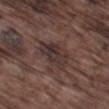workup: imaged on a skin check; not biopsied
patient: male, roughly 75 years of age
automated lesion analysis: an area of roughly 11 mm², an outline eccentricity of about 0.75 (0 = round, 1 = elongated), and two-axis asymmetry of about 0.25; an average lesion color of about L≈32 a*≈15 b*≈17 (CIELAB), a lesion–skin lightness drop of about 7, and a lesion-to-skin contrast of about 8 (normalized; higher = more distinct); a classifier nevus-likeness of about 0/100
lesion size: ≈5 mm
image source: 15 mm crop, total-body photography
tile lighting: white-light illumination
body site: the left thigh